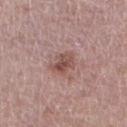  biopsy_status: not biopsied; imaged during a skin examination
  image:
    source: total-body photography crop
    field_of_view_mm: 15
  lesion_size:
    long_diameter_mm_approx: 3.5
  patient:
    sex: male
    age_approx: 75
  lighting: white-light
  site: right thigh